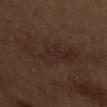Notes:
* biopsy status · catalogued during a skin exam; not biopsied